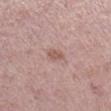diameter: ≈2.5 mm | lighting: white-light | imaging modality: total-body-photography crop, ~15 mm field of view | anatomic site: the right lower leg | patient: female, aged around 40.